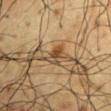Assessment:
No biopsy was performed on this lesion — it was imaged during a full skin examination and was not determined to be concerning.
Acquisition and patient details:
A lesion tile, about 15 mm wide, cut from a 3D total-body photograph. This is a cross-polarized tile. On the arm. A male subject, approximately 60 years of age. Measured at roughly 2.5 mm in maximum diameter.